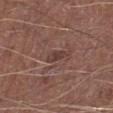Recorded during total-body skin imaging; not selected for excision or biopsy. The subject is a male aged 73–77. A roughly 15 mm field-of-view crop from a total-body skin photograph. On the left lower leg. Imaged with white-light lighting.